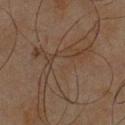Assessment:
This lesion was catalogued during total-body skin photography and was not selected for biopsy.
Acquisition and patient details:
A 15 mm close-up extracted from a 3D total-body photography capture. The lesion is on the chest. The patient is a male aged 43–47.